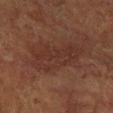Recorded during total-body skin imaging; not selected for excision or biopsy. The tile uses cross-polarized illumination. The lesion is located on the leg. A close-up tile cropped from a whole-body skin photograph, about 15 mm across. A male patient, roughly 80 years of age. Automated tile analysis of the lesion measured a footprint of about 18 mm², a shape eccentricity near 0.9, and a shape-asymmetry score of about 0.3 (0 = symmetric). The software also gave a nevus-likeness score of about 0/100.